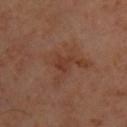| feature | finding |
|---|---|
| follow-up | catalogued during a skin exam; not biopsied |
| site | the upper back |
| patient | male, aged 63 to 67 |
| image | total-body-photography crop, ~15 mm field of view |